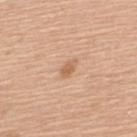Imaged during a routine full-body skin examination; the lesion was not biopsied and no histopathology is available. A female subject about 60 years old. A roughly 15 mm field-of-view crop from a total-body skin photograph. Captured under white-light illumination. Automated image analysis of the tile measured an automated nevus-likeness rating near 25 out of 100 and a detector confidence of about 100 out of 100 that the crop contains a lesion. The recorded lesion diameter is about 2.5 mm. Located on the back.